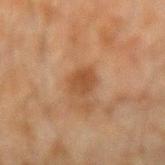Notes:
* biopsy status · imaged on a skin check; not biopsied
* lesion diameter · ~3 mm (longest diameter)
* image · 15 mm crop, total-body photography
* patient · male, roughly 45 years of age
* anatomic site · the arm
* tile lighting · cross-polarized
* automated metrics · border irregularity of about 1.5 on a 0–10 scale, a color-variation rating of about 2/10, and peripheral color asymmetry of about 1